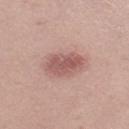follow-up = no biopsy performed (imaged during a skin exam) | subject = female, approximately 20 years of age | size = ~5 mm (longest diameter) | image source = ~15 mm crop, total-body skin-cancer survey | body site = the leg | tile lighting = white-light.